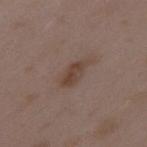notes: imaged on a skin check; not biopsied | image source: ~15 mm tile from a whole-body skin photo | subject: female, approximately 35 years of age | site: the upper back.